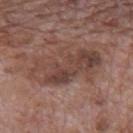Assessment: No biopsy was performed on this lesion — it was imaged during a full skin examination and was not determined to be concerning. Image and clinical context: Approximately 9 mm at its widest. The patient is a male in their 70s. The lesion is on the mid back. A 15 mm crop from a total-body photograph taken for skin-cancer surveillance.